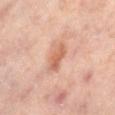The lesion was tiled from a total-body skin photograph and was not biopsied.
Imaged with cross-polarized lighting.
Cropped from a whole-body photographic skin survey; the tile spans about 15 mm.
A female patient, about 50 years old.
From the left lower leg.
An algorithmic analysis of the crop reported a lesion area of about 5 mm² and an outline eccentricity of about 0.85 (0 = round, 1 = elongated). It also reported an average lesion color of about L≈60 a*≈24 b*≈32 (CIELAB) and a lesion–skin lightness drop of about 10.